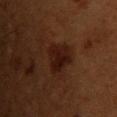notes: imaged on a skin check; not biopsied
subject: male, about 50 years old
location: the chest
image source: 15 mm crop, total-body photography
image-analysis metrics: an automated nevus-likeness rating near 55 out of 100 and a lesion-detection confidence of about 100/100
tile lighting: cross-polarized